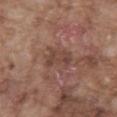Q: Is there a histopathology result?
A: catalogued during a skin exam; not biopsied
Q: How large is the lesion?
A: ≈3.5 mm
Q: What is the anatomic site?
A: the abdomen
Q: What are the patient's age and sex?
A: male, about 75 years old
Q: Automated lesion metrics?
A: a footprint of about 7 mm², an outline eccentricity of about 0.8 (0 = round, 1 = elongated), and a symmetry-axis asymmetry near 0.3; a mean CIELAB color near L≈43 a*≈19 b*≈24; border irregularity of about 3.5 on a 0–10 scale, a color-variation rating of about 3/10, and radial color variation of about 1
Q: What is the imaging modality?
A: 15 mm crop, total-body photography
Q: What lighting was used for the tile?
A: white-light illumination